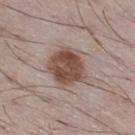workup: no biopsy performed (imaged during a skin exam); image: total-body-photography crop, ~15 mm field of view; anatomic site: the leg; size: about 4.5 mm; patient: male, about 50 years old; tile lighting: white-light illumination.